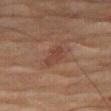Recorded during total-body skin imaging; not selected for excision or biopsy.
A male patient in their mid-80s or older.
From the right thigh.
A roughly 15 mm field-of-view crop from a total-body skin photograph.